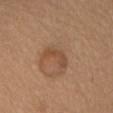No biopsy was performed on this lesion — it was imaged during a full skin examination and was not determined to be concerning. A female patient, aged approximately 60. About 3.5 mm across. A 15 mm crop from a total-body photograph taken for skin-cancer surveillance. On the head or neck.